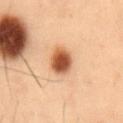imaging modality — 15 mm crop, total-body photography
subject — male, aged around 55
image-analysis metrics — a lesion color around L≈45 a*≈22 b*≈32 in CIELAB, roughly 16 lightness units darker than nearby skin, and a normalized border contrast of about 12
lighting — cross-polarized illumination
diameter — ~3 mm (longest diameter)
body site — the front of the torso